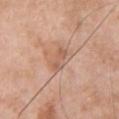The lesion was photographed on a routine skin check and not biopsied; there is no pathology result. Imaged with white-light lighting. A 15 mm close-up tile from a total-body photography series done for melanoma screening. Located on the chest. The recorded lesion diameter is about 3 mm. The total-body-photography lesion software estimated a lesion area of about 4 mm², an eccentricity of roughly 0.9, and two-axis asymmetry of about 0.25. The software also gave border irregularity of about 4 on a 0–10 scale and a peripheral color-asymmetry measure near 0. And it measured an automated nevus-likeness rating near 0 out of 100 and a lesion-detection confidence of about 100/100. A male patient, aged around 55.